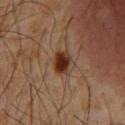No biopsy was performed on this lesion — it was imaged during a full skin examination and was not determined to be concerning.
Measured at roughly 2.5 mm in maximum diameter.
On the chest.
A male subject aged 58 to 62.
This image is a 15 mm lesion crop taken from a total-body photograph.
Captured under cross-polarized illumination.